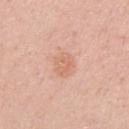| field | value |
|---|---|
| notes | no biopsy performed (imaged during a skin exam) |
| size | about 3 mm |
| anatomic site | the right upper arm |
| patient | female, approximately 30 years of age |
| imaging modality | ~15 mm tile from a whole-body skin photo |
| TBP lesion metrics | internal color variation of about 2.5 on a 0–10 scale; a classifier nevus-likeness of about 0/100 |
| illumination | white-light |